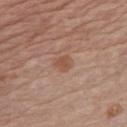biopsy_status: not biopsied; imaged during a skin examination
automated_metrics:
  area_mm2_approx: 4.0
  eccentricity: 0.7
  cielab_L: 52
  cielab_a: 21
  cielab_b: 29
  vs_skin_contrast_norm: 6.0
  border_irregularity_0_10: 2.0
  color_variation_0_10: 1.5
  peripheral_color_asymmetry: 0.5
image:
  source: total-body photography crop
  field_of_view_mm: 15
lesion_size:
  long_diameter_mm_approx: 2.5
lighting: white-light
site: chest
patient:
  sex: male
  age_approx: 70